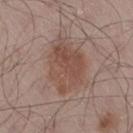biopsy status: imaged on a skin check; not biopsied
tile lighting: white-light
patient: male, aged 53–57
image-analysis metrics: a footprint of about 18 mm², an eccentricity of roughly 0.7, and a shape-asymmetry score of about 0.3 (0 = symmetric); a border-irregularity rating of about 4/10, a color-variation rating of about 4/10, and a peripheral color-asymmetry measure near 1.5; an automated nevus-likeness rating near 60 out of 100 and a lesion-detection confidence of about 100/100
image source: ~15 mm tile from a whole-body skin photo
location: the right thigh